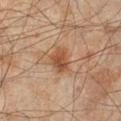Impression: No biopsy was performed on this lesion — it was imaged during a full skin examination and was not determined to be concerning.